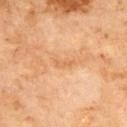Findings:
• workup — no biopsy performed (imaged during a skin exam)
• lighting — cross-polarized
• body site — the upper back
• size — ~2.5 mm (longest diameter)
• image source — 15 mm crop, total-body photography
• subject — male, aged 68–72